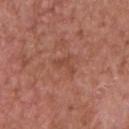Background:
A 15 mm close-up tile from a total-body photography series done for melanoma screening. A male subject, about 65 years old. Measured at roughly 3 mm in maximum diameter. Automated tile analysis of the lesion measured a border-irregularity index near 7.5/10, a within-lesion color-variation index near 0/10, and a peripheral color-asymmetry measure near 0. The software also gave a classifier nevus-likeness of about 0/100 and lesion-presence confidence of about 100/100. The lesion is located on the chest. Captured under white-light illumination.